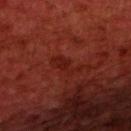Notes:
* workup · imaged on a skin check; not biopsied
* acquisition · ~15 mm crop, total-body skin-cancer survey
* site · the upper back
* patient · male, aged 58 to 62
* lighting · cross-polarized